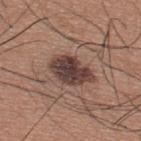Notes:
– biopsy status · total-body-photography surveillance lesion; no biopsy
– body site · the upper back
– lesion size · ~5 mm (longest diameter)
– subject · male, aged 33–37
– automated metrics · a footprint of about 12 mm², an outline eccentricity of about 0.75 (0 = round, 1 = elongated), and two-axis asymmetry of about 0.2
– acquisition · 15 mm crop, total-body photography